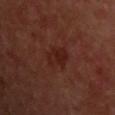workup: no biopsy performed (imaged during a skin exam) | body site: the upper back | subject: male, roughly 50 years of age | automated lesion analysis: a mean CIELAB color near L≈22 a*≈23 b*≈23, about 5 CIELAB-L* units darker than the surrounding skin, and a normalized border contrast of about 6.5; a classifier nevus-likeness of about 60/100 and lesion-presence confidence of about 100/100 | image source: 15 mm crop, total-body photography | tile lighting: cross-polarized | lesion diameter: about 3.5 mm.